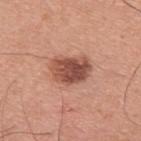biopsy status: catalogued during a skin exam; not biopsied
location: the upper back
size: ~4.5 mm (longest diameter)
acquisition: total-body-photography crop, ~15 mm field of view
subject: male, roughly 30 years of age
tile lighting: white-light